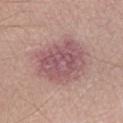Part of a total-body skin-imaging series; this lesion was reviewed on a skin check and was not flagged for biopsy. A lesion tile, about 15 mm wide, cut from a 3D total-body photograph. A male patient approximately 40 years of age. On the right lower leg. The lesion-visualizer software estimated an eccentricity of roughly 0.35 and a symmetry-axis asymmetry near 0.2. It also reported about 10 CIELAB-L* units darker than the surrounding skin and a lesion-to-skin contrast of about 8 (normalized; higher = more distinct). The software also gave border irregularity of about 2.5 on a 0–10 scale and peripheral color asymmetry of about 1.5. The software also gave an automated nevus-likeness rating near 5 out of 100 and a lesion-detection confidence of about 100/100.